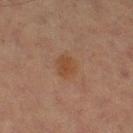Assessment: This lesion was catalogued during total-body skin photography and was not selected for biopsy. Context: Cropped from a whole-body photographic skin survey; the tile spans about 15 mm. On the leg. Automated tile analysis of the lesion measured border irregularity of about 2 on a 0–10 scale, internal color variation of about 2 on a 0–10 scale, and a peripheral color-asymmetry measure near 0.5. It also reported a detector confidence of about 100 out of 100 that the crop contains a lesion. A male subject, aged approximately 70.